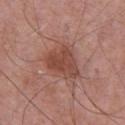{"biopsy_status": "not biopsied; imaged during a skin examination"}